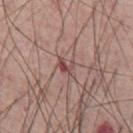Captured during whole-body skin photography for melanoma surveillance; the lesion was not biopsied. Imaged with white-light lighting. A male subject aged around 55. Longest diameter approximately 2.5 mm. The lesion is located on the front of the torso. Automated image analysis of the tile measured an area of roughly 3.5 mm² and a shape eccentricity near 0.7. The software also gave an average lesion color of about L≈49 a*≈21 b*≈20 (CIELAB), about 9 CIELAB-L* units darker than the surrounding skin, and a lesion-to-skin contrast of about 7 (normalized; higher = more distinct). The software also gave a classifier nevus-likeness of about 0/100. A roughly 15 mm field-of-view crop from a total-body skin photograph.